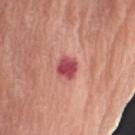subject: male, approximately 80 years of age; location: the right upper arm; imaging modality: 15 mm crop, total-body photography.